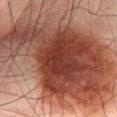  biopsy_status: not biopsied; imaged during a skin examination
  site: lower back
  image:
    source: total-body photography crop
    field_of_view_mm: 15
  patient:
    sex: male
    age_approx: 65
  lesion_size:
    long_diameter_mm_approx: 16.5